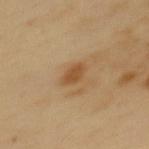Q: Was this lesion biopsied?
A: no biopsy performed (imaged during a skin exam)
Q: Where on the body is the lesion?
A: the back
Q: What kind of image is this?
A: 15 mm crop, total-body photography
Q: Patient demographics?
A: female, approximately 50 years of age
Q: What lighting was used for the tile?
A: cross-polarized
Q: Lesion size?
A: about 2.5 mm